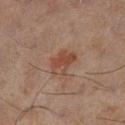Clinical summary: From the left lower leg. This is a cross-polarized tile. This image is a 15 mm lesion crop taken from a total-body photograph. An algorithmic analysis of the crop reported an area of roughly 6.5 mm², a shape eccentricity near 0.7, and two-axis asymmetry of about 0.25. The software also gave a lesion color around L≈36 a*≈18 b*≈23 in CIELAB, about 7 CIELAB-L* units darker than the surrounding skin, and a normalized lesion–skin contrast near 6.5. It also reported a classifier nevus-likeness of about 15/100. Measured at roughly 3.5 mm in maximum diameter. The patient is a male in their mid-40s.